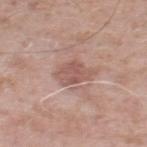The lesion was photographed on a routine skin check and not biopsied; there is no pathology result.
Measured at roughly 4 mm in maximum diameter.
A male patient roughly 60 years of age.
Cropped from a total-body skin-imaging series; the visible field is about 15 mm.
From the leg.
This is a white-light tile.